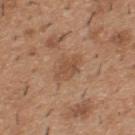The lesion was photographed on a routine skin check and not biopsied; there is no pathology result.
This image is a 15 mm lesion crop taken from a total-body photograph.
The lesion's longest dimension is about 3.5 mm.
The lesion-visualizer software estimated a border-irregularity index near 3/10, a within-lesion color-variation index near 2/10, and peripheral color asymmetry of about 0.5. The analysis additionally found lesion-presence confidence of about 100/100.
The lesion is on the upper back.
This is a white-light tile.
A male patient, roughly 40 years of age.